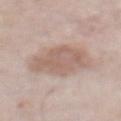Notes:
- notes · imaged on a skin check; not biopsied
- patient · male, roughly 75 years of age
- automated metrics · an average lesion color of about L≈60 a*≈16 b*≈24 (CIELAB) and a lesion-to-skin contrast of about 6 (normalized; higher = more distinct); a border-irregularity index near 3/10 and a color-variation rating of about 2.5/10
- illumination · white-light
- body site · the abdomen
- image source · total-body-photography crop, ~15 mm field of view
- lesion size · ~6 mm (longest diameter)